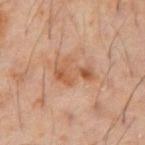Notes:
– biopsy status · catalogued during a skin exam; not biopsied
– anatomic site · the abdomen
– patient · male, roughly 60 years of age
– image source · 15 mm crop, total-body photography
– TBP lesion metrics · a footprint of about 9 mm², an outline eccentricity of about 0.8 (0 = round, 1 = elongated), and two-axis asymmetry of about 0.45; an average lesion color of about L≈52 a*≈21 b*≈32 (CIELAB), roughly 8 lightness units darker than nearby skin, and a lesion-to-skin contrast of about 6.5 (normalized; higher = more distinct); internal color variation of about 4 on a 0–10 scale and peripheral color asymmetry of about 1; a nevus-likeness score of about 0/100 and lesion-presence confidence of about 100/100